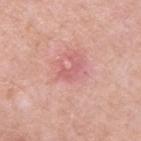<case>
  <site>upper back</site>
  <patient>
    <sex>male</sex>
    <age_approx>55</age_approx>
  </patient>
  <lighting>white-light</lighting>
  <lesion_size>
    <long_diameter_mm_approx>2.5</long_diameter_mm_approx>
  </lesion_size>
  <image>
    <source>total-body photography crop</source>
    <field_of_view_mm>15</field_of_view_mm>
  </image>
</case>